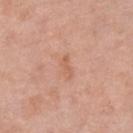Captured during whole-body skin photography for melanoma surveillance; the lesion was not biopsied.
Located on the left lower leg.
Cropped from a whole-body photographic skin survey; the tile spans about 15 mm.
A female patient, aged around 70.